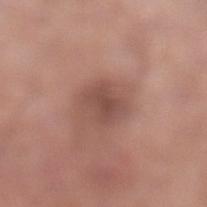Captured during whole-body skin photography for melanoma surveillance; the lesion was not biopsied.